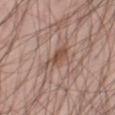Recorded during total-body skin imaging; not selected for excision or biopsy. From the leg. About 4 mm across. The total-body-photography lesion software estimated a lesion area of about 6 mm², a shape eccentricity near 0.9, and a symmetry-axis asymmetry near 0.45. It also reported about 9 CIELAB-L* units darker than the surrounding skin and a normalized border contrast of about 7. This is a white-light tile. A 15 mm close-up extracted from a 3D total-body photography capture. The patient is a male aged around 45.